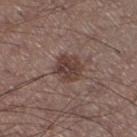The recorded lesion diameter is about 3.5 mm. The lesion is located on the leg. The patient is a male aged 53–57. A 15 mm close-up extracted from a 3D total-body photography capture.